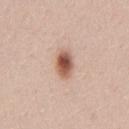Impression:
The lesion was photographed on a routine skin check and not biopsied; there is no pathology result.
Image and clinical context:
A region of skin cropped from a whole-body photographic capture, roughly 15 mm wide. A female patient, aged around 30. The lesion is located on the lower back. The recorded lesion diameter is about 3 mm. Imaged with white-light lighting.